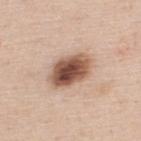Q: Who is the patient?
A: male, aged approximately 45
Q: Where on the body is the lesion?
A: the upper back
Q: What kind of image is this?
A: ~15 mm tile from a whole-body skin photo